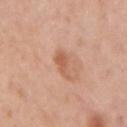Q: Where on the body is the lesion?
A: the right upper arm
Q: What kind of image is this?
A: ~15 mm crop, total-body skin-cancer survey
Q: Who is the patient?
A: female, approximately 45 years of age
Q: Illumination type?
A: white-light illumination
Q: How large is the lesion?
A: ~3 mm (longest diameter)